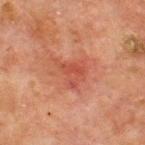Recorded during total-body skin imaging; not selected for excision or biopsy.
The lesion's longest dimension is about 4 mm.
The total-body-photography lesion software estimated a mean CIELAB color near L≈41 a*≈26 b*≈28, roughly 6 lightness units darker than nearby skin, and a lesion-to-skin contrast of about 5.5 (normalized; higher = more distinct). The software also gave a border-irregularity index near 5.5/10 and internal color variation of about 3.5 on a 0–10 scale.
The patient is a male in their mid- to late 60s.
Captured under cross-polarized illumination.
A 15 mm close-up extracted from a 3D total-body photography capture.
On the chest.